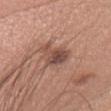The lesion was tiled from a total-body skin photograph and was not biopsied. A close-up tile cropped from a whole-body skin photograph, about 15 mm across. A female patient, aged around 35. The lesion is on the head or neck. Imaged with white-light lighting. The lesion's longest dimension is about 4 mm. Automated tile analysis of the lesion measured a nevus-likeness score of about 30/100 and a lesion-detection confidence of about 100/100.